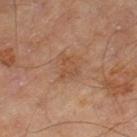Findings:
• follow-up · catalogued during a skin exam; not biopsied
• tile lighting · cross-polarized illumination
• lesion size · ~2.5 mm (longest diameter)
• site · the leg
• imaging modality · ~15 mm tile from a whole-body skin photo
• subject · male, in their 70s